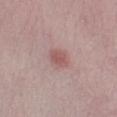The lesion was tiled from a total-body skin photograph and was not biopsied.
The lesion-visualizer software estimated a border-irregularity rating of about 1.5/10, a color-variation rating of about 1.5/10, and peripheral color asymmetry of about 0.5. It also reported a classifier nevus-likeness of about 65/100 and a lesion-detection confidence of about 100/100.
The patient is a male aged approximately 50.
From the right lower leg.
This image is a 15 mm lesion crop taken from a total-body photograph.